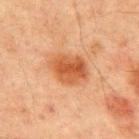Captured during whole-body skin photography for melanoma surveillance; the lesion was not biopsied. From the front of the torso. The recorded lesion diameter is about 4.5 mm. Imaged with cross-polarized lighting. A 15 mm close-up extracted from a 3D total-body photography capture. The patient is a male aged around 65.